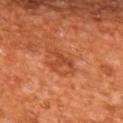<tbp_lesion>
<biopsy_status>not biopsied; imaged during a skin examination</biopsy_status>
<automated_metrics>
  <cielab_L>44</cielab_L>
  <cielab_a>31</cielab_a>
  <cielab_b>38</cielab_b>
  <vs_skin_contrast_norm>6.0</vs_skin_contrast_norm>
  <border_irregularity_0_10>7.0</border_irregularity_0_10>
  <color_variation_0_10>1.0</color_variation_0_10>
  <peripheral_color_asymmetry>0.0</peripheral_color_asymmetry>
</automated_metrics>
<site>back</site>
<patient>
  <sex>male</sex>
  <age_approx>65</age_approx>
</patient>
<lighting>cross-polarized</lighting>
<lesion_size>
  <long_diameter_mm_approx>3.0</long_diameter_mm_approx>
</lesion_size>
<image>
  <source>total-body photography crop</source>
  <field_of_view_mm>15</field_of_view_mm>
</image>
</tbp_lesion>